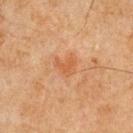notes = catalogued during a skin exam; not biopsied
tile lighting = cross-polarized
body site = the chest
image = 15 mm crop, total-body photography
lesion diameter = about 2.5 mm
patient = male, roughly 65 years of age